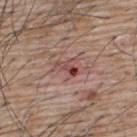biopsy status: total-body-photography surveillance lesion; no biopsy
imaging modality: ~15 mm crop, total-body skin-cancer survey
size: about 3 mm
body site: the upper back
patient: male, aged around 65